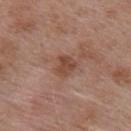biopsy status: no biopsy performed (imaged during a skin exam).